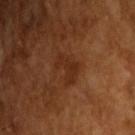• workup: total-body-photography surveillance lesion; no biopsy